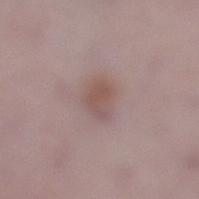anatomic site = the right lower leg
image = 15 mm crop, total-body photography
patient = male, about 50 years old